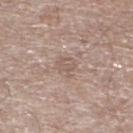biopsy_status: not biopsied; imaged during a skin examination
image:
  source: total-body photography crop
  field_of_view_mm: 15
site: left lower leg
lighting: white-light
lesion_size:
  long_diameter_mm_approx: 3.0
automated_metrics:
  border_irregularity_0_10: 4.0
  color_variation_0_10: 2.0
  peripheral_color_asymmetry: 0.5
  nevus_likeness_0_100: 0
  lesion_detection_confidence_0_100: 95
patient:
  sex: male
  age_approx: 65